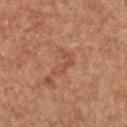{"biopsy_status": "not biopsied; imaged during a skin examination", "lighting": "white-light", "patient": {"sex": "female", "age_approx": 40}, "lesion_size": {"long_diameter_mm_approx": 3.5}, "image": {"source": "total-body photography crop", "field_of_view_mm": 15}, "site": "chest"}